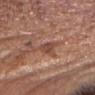• site — the head or neck
• lesion diameter — about 2.5 mm
• patient — male, about 75 years old
• image — ~15 mm tile from a whole-body skin photo